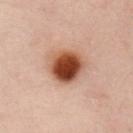notes = imaged on a skin check; not biopsied
diameter = ~4 mm (longest diameter)
tile lighting = cross-polarized illumination
patient = female, about 55 years old
location = the chest
image = 15 mm crop, total-body photography